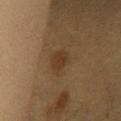Clinical impression: Captured during whole-body skin photography for melanoma surveillance; the lesion was not biopsied. Acquisition and patient details: The total-body-photography lesion software estimated a border-irregularity rating of about 2.5/10 and a color-variation rating of about 1.5/10. It also reported a nevus-likeness score of about 80/100. This is a cross-polarized tile. A 15 mm close-up extracted from a 3D total-body photography capture. The patient is a female about 40 years old. The lesion's longest dimension is about 3.5 mm. The lesion is located on the chest.